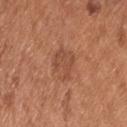Recorded during total-body skin imaging; not selected for excision or biopsy. The lesion's longest dimension is about 3.5 mm. The lesion is located on the upper back. A male patient aged approximately 55. Cropped from a whole-body photographic skin survey; the tile spans about 15 mm. An algorithmic analysis of the crop reported an average lesion color of about L≈49 a*≈24 b*≈32 (CIELAB), about 7 CIELAB-L* units darker than the surrounding skin, and a lesion-to-skin contrast of about 5.5 (normalized; higher = more distinct). It also reported border irregularity of about 3 on a 0–10 scale, a color-variation rating of about 2/10, and a peripheral color-asymmetry measure near 0.5. The tile uses white-light illumination.